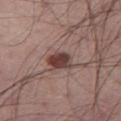TBP lesion metrics=a lesion color around L≈40 a*≈19 b*≈21 in CIELAB, a lesion–skin lightness drop of about 14, and a normalized border contrast of about 11; a border-irregularity rating of about 2.5/10 and a within-lesion color-variation index near 4/10; a lesion-detection confidence of about 100/100 | patient=male, roughly 55 years of age | body site=the left thigh | image source=~15 mm crop, total-body skin-cancer survey | lesion size=~3.5 mm (longest diameter).